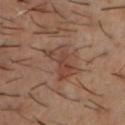The lesion was tiled from a total-body skin photograph and was not biopsied.